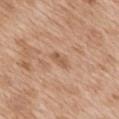A female subject aged around 40. A 15 mm close-up extracted from a 3D total-body photography capture. The lesion is on the mid back. The tile uses white-light illumination. Approximately 2.5 mm at its widest. Automated image analysis of the tile measured an average lesion color of about L≈57 a*≈20 b*≈33 (CIELAB), about 9 CIELAB-L* units darker than the surrounding skin, and a normalized border contrast of about 6. The software also gave a border-irregularity index near 3/10, internal color variation of about 0 on a 0–10 scale, and radial color variation of about 0.